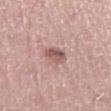A male subject, aged 58 to 62.
The lesion is on the left thigh.
Imaged with white-light lighting.
The lesion's longest dimension is about 2.5 mm.
A 15 mm crop from a total-body photograph taken for skin-cancer surveillance.